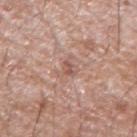Q: Was this lesion biopsied?
A: no biopsy performed (imaged during a skin exam)
Q: What is the imaging modality?
A: ~15 mm tile from a whole-body skin photo
Q: Where on the body is the lesion?
A: the arm
Q: What are the patient's age and sex?
A: male, in their 60s
Q: How was the tile lit?
A: white-light